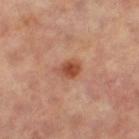Impression:
Imaged during a routine full-body skin examination; the lesion was not biopsied and no histopathology is available.
Image and clinical context:
A female patient, roughly 65 years of age. A 15 mm close-up extracted from a 3D total-body photography capture. From the right leg. The recorded lesion diameter is about 3 mm. This is a cross-polarized tile.